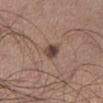follow-up: total-body-photography surveillance lesion; no biopsy
lesion diameter: about 3 mm
site: the right lower leg
patient: male, roughly 25 years of age
image: ~15 mm crop, total-body skin-cancer survey
tile lighting: white-light